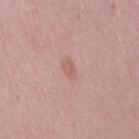The lesion was photographed on a routine skin check and not biopsied; there is no pathology result.
Imaged with white-light lighting.
On the lower back.
A female subject, aged around 15.
Cropped from a whole-body photographic skin survey; the tile spans about 15 mm.
Automated image analysis of the tile measured an eccentricity of roughly 0.8 and a symmetry-axis asymmetry near 0.3.